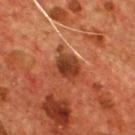The lesion was photographed on a routine skin check and not biopsied; there is no pathology result. The tile uses cross-polarized illumination. About 4.5 mm across. On the chest. The subject is a male about 55 years old. The total-body-photography lesion software estimated a border-irregularity index near 3.5/10, a within-lesion color-variation index near 4.5/10, and peripheral color asymmetry of about 1.5. This image is a 15 mm lesion crop taken from a total-body photograph.